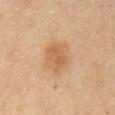lesion diameter: ≈4 mm | tile lighting: cross-polarized | subject: male, aged around 85 or older | imaging modality: ~15 mm crop, total-body skin-cancer survey | image-analysis metrics: a lesion area of about 12 mm², an eccentricity of roughly 0.6, and a shape-asymmetry score of about 0.2 (0 = symmetric); a lesion color around L≈59 a*≈19 b*≈36 in CIELAB, about 8 CIELAB-L* units darker than the surrounding skin, and a lesion-to-skin contrast of about 6 (normalized; higher = more distinct); a border-irregularity rating of about 2/10, a within-lesion color-variation index near 3/10, and radial color variation of about 1 | site: the chest.